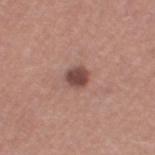The total-body-photography lesion software estimated a mean CIELAB color near L≈46 a*≈21 b*≈23. A close-up tile cropped from a whole-body skin photograph, about 15 mm across. From the leg. This is a white-light tile. A female subject aged around 60.